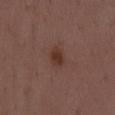Clinical impression:
Recorded during total-body skin imaging; not selected for excision or biopsy.
Background:
The subject is a male aged 38 to 42. The lesion's longest dimension is about 2.5 mm. Located on the lower back. This is a white-light tile. Cropped from a total-body skin-imaging series; the visible field is about 15 mm.